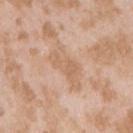Impression: The lesion was photographed on a routine skin check and not biopsied; there is no pathology result. Acquisition and patient details: The patient is a female in their mid- to late 20s. This is a white-light tile. A region of skin cropped from a whole-body photographic capture, roughly 15 mm wide. The lesion is on the right upper arm.